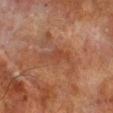Clinical impression:
Recorded during total-body skin imaging; not selected for excision or biopsy.
Context:
A region of skin cropped from a whole-body photographic capture, roughly 15 mm wide. The subject is a male roughly 70 years of age. On the right lower leg.